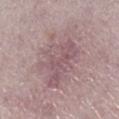| key | value |
|---|---|
| automated metrics | an area of roughly 30 mm² and a shape eccentricity near 0.6; a detector confidence of about 70 out of 100 that the crop contains a lesion |
| lesion diameter | ≈7 mm |
| lighting | white-light illumination |
| patient | female, about 60 years old |
| site | the right lower leg |
| image | ~15 mm crop, total-body skin-cancer survey |